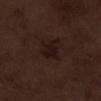The lesion was tiled from a total-body skin photograph and was not biopsied. From the left lower leg. A roughly 15 mm field-of-view crop from a total-body skin photograph. A male subject aged around 70.